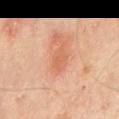Clinical impression: No biopsy was performed on this lesion — it was imaged during a full skin examination and was not determined to be concerning. Context: Imaged with cross-polarized lighting. The lesion is on the chest. The lesion-visualizer software estimated a footprint of about 3 mm², an outline eccentricity of about 0.8 (0 = round, 1 = elongated), and two-axis asymmetry of about 0.45. And it measured a lesion color around L≈60 a*≈27 b*≈36 in CIELAB, about 7 CIELAB-L* units darker than the surrounding skin, and a normalized border contrast of about 5. And it measured a border-irregularity rating of about 4.5/10, a color-variation rating of about 1/10, and peripheral color asymmetry of about 0.5. A close-up tile cropped from a whole-body skin photograph, about 15 mm across. The recorded lesion diameter is about 2.5 mm. The patient is a male aged approximately 65.